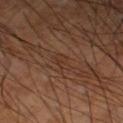Q: Was a biopsy performed?
A: total-body-photography surveillance lesion; no biopsy
Q: What are the patient's age and sex?
A: male, approximately 60 years of age
Q: What kind of image is this?
A: ~15 mm tile from a whole-body skin photo
Q: Lesion location?
A: the left thigh